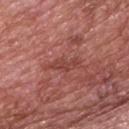Case summary:
– follow-up — imaged on a skin check; not biopsied
– subject — male, aged around 70
– lesion size — ≈3.5 mm
– TBP lesion metrics — a lesion area of about 4 mm², a shape eccentricity near 0.9, and a symmetry-axis asymmetry near 0.35; roughly 7 lightness units darker than nearby skin and a lesion-to-skin contrast of about 6 (normalized; higher = more distinct); a color-variation rating of about 1/10 and radial color variation of about 0.5
– location — the back
– imaging modality — ~15 mm crop, total-body skin-cancer survey